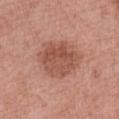Impression: Recorded during total-body skin imaging; not selected for excision or biopsy. Context: This image is a 15 mm lesion crop taken from a total-body photograph. On the left thigh. A female subject aged approximately 70. Captured under white-light illumination.